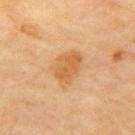| key | value |
|---|---|
| workup | no biopsy performed (imaged during a skin exam) |
| lighting | cross-polarized |
| acquisition | ~15 mm crop, total-body skin-cancer survey |
| size | about 4.5 mm |
| anatomic site | the chest |
| subject | male, in their mid-80s or older |